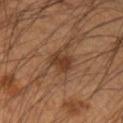workup — imaged on a skin check; not biopsied
anatomic site — the left forearm
automated lesion analysis — an automated nevus-likeness rating near 80 out of 100
tile lighting — cross-polarized illumination
image source — ~15 mm tile from a whole-body skin photo
subject — male, in their mid- to late 60s